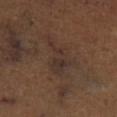The lesion is on the left lower leg. The patient is a female aged 63 to 67. Imaged with cross-polarized lighting. A lesion tile, about 15 mm wide, cut from a 3D total-body photograph.